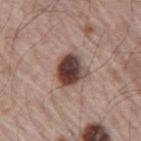Part of a total-body skin-imaging series; this lesion was reviewed on a skin check and was not flagged for biopsy.
The lesion-visualizer software estimated a footprint of about 11 mm², an eccentricity of roughly 0.45, and a shape-asymmetry score of about 0.2 (0 = symmetric). The analysis additionally found an average lesion color of about L≈42 a*≈18 b*≈21 (CIELAB), about 18 CIELAB-L* units darker than the surrounding skin, and a normalized lesion–skin contrast near 13.5. It also reported internal color variation of about 9 on a 0–10 scale.
The patient is a male aged 63–67.
The lesion is on the arm.
Longest diameter approximately 4 mm.
A roughly 15 mm field-of-view crop from a total-body skin photograph.